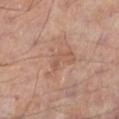A 15 mm close-up tile from a total-body photography series done for melanoma screening. The subject is a male in their mid- to late 50s. Located on the leg. About 3 mm across.